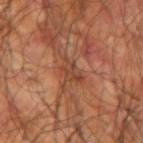biopsy status: imaged on a skin check; not biopsied
lighting: cross-polarized illumination
patient: male, about 60 years old
imaging modality: ~15 mm tile from a whole-body skin photo
anatomic site: the arm